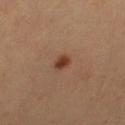Q: Is there a histopathology result?
A: total-body-photography surveillance lesion; no biopsy
Q: Where on the body is the lesion?
A: the left thigh
Q: What are the patient's age and sex?
A: female, in their mid- to late 50s
Q: What kind of image is this?
A: ~15 mm tile from a whole-body skin photo